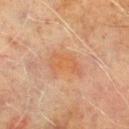Assessment: This lesion was catalogued during total-body skin photography and was not selected for biopsy. Context: Cropped from a whole-body photographic skin survey; the tile spans about 15 mm. The lesion's longest dimension is about 4.5 mm. A male patient, aged 68 to 72. On the left lower leg.